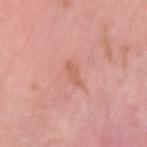Impression:
This lesion was catalogued during total-body skin photography and was not selected for biopsy.
Clinical summary:
The lesion is on the left upper arm. The patient is a male aged 23–27. A roughly 15 mm field-of-view crop from a total-body skin photograph.